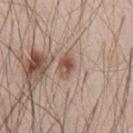Impression:
The lesion was photographed on a routine skin check and not biopsied; there is no pathology result.
Background:
The total-body-photography lesion software estimated an average lesion color of about L≈52 a*≈18 b*≈27 (CIELAB), about 11 CIELAB-L* units darker than the surrounding skin, and a normalized border contrast of about 8. This is a white-light tile. A male subject, about 45 years old. The recorded lesion diameter is about 3 mm. On the chest. A 15 mm close-up tile from a total-body photography series done for melanoma screening.